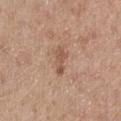notes: catalogued during a skin exam; not biopsied | subject: female, approximately 40 years of age | image: 15 mm crop, total-body photography | site: the left forearm.